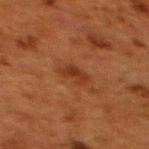Clinical impression:
The lesion was tiled from a total-body skin photograph and was not biopsied.
Background:
From the upper back. About 3 mm across. The tile uses cross-polarized illumination. An algorithmic analysis of the crop reported a border-irregularity rating of about 3.5/10, internal color variation of about 2.5 on a 0–10 scale, and radial color variation of about 0.5. A roughly 15 mm field-of-view crop from a total-body skin photograph. The subject is a female aged approximately 50.